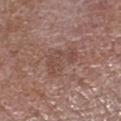workup: catalogued during a skin exam; not biopsied | anatomic site: the right forearm | image: total-body-photography crop, ~15 mm field of view | lesion size: ≈4.5 mm | TBP lesion metrics: a mean CIELAB color near L≈47 a*≈19 b*≈23, roughly 6 lightness units darker than nearby skin, and a lesion-to-skin contrast of about 5 (normalized; higher = more distinct) | tile lighting: white-light | patient: male, about 65 years old.